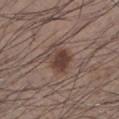Impression:
The lesion was tiled from a total-body skin photograph and was not biopsied.
Clinical summary:
A lesion tile, about 15 mm wide, cut from a 3D total-body photograph. On the left lower leg. The subject is a male in their mid- to late 30s. Measured at roughly 4 mm in maximum diameter. Imaged with white-light lighting.